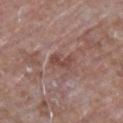No biopsy was performed on this lesion — it was imaged during a full skin examination and was not determined to be concerning. About 2.5 mm across. From the upper back. A roughly 15 mm field-of-view crop from a total-body skin photograph. The total-body-photography lesion software estimated an automated nevus-likeness rating near 0 out of 100 and lesion-presence confidence of about 95/100. The tile uses white-light illumination. A male patient, in their mid- to late 60s.